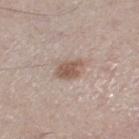The lesion was tiled from a total-body skin photograph and was not biopsied. The total-body-photography lesion software estimated a footprint of about 5.5 mm² and an eccentricity of roughly 0.8. The analysis additionally found an average lesion color of about L≈55 a*≈17 b*≈25 (CIELAB) and a normalized lesion–skin contrast near 8.5. The software also gave a nevus-likeness score of about 85/100. Approximately 3.5 mm at its widest. From the right lower leg. A male patient aged 58 to 62. The tile uses white-light illumination. A 15 mm close-up extracted from a 3D total-body photography capture.